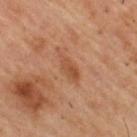The lesion was photographed on a routine skin check and not biopsied; there is no pathology result.
A male patient, in their mid- to late 50s.
The total-body-photography lesion software estimated a lesion area of about 5.5 mm², a shape eccentricity near 0.9, and a symmetry-axis asymmetry near 0.2. The software also gave a mean CIELAB color near L≈49 a*≈23 b*≈34, about 8 CIELAB-L* units darker than the surrounding skin, and a normalized lesion–skin contrast near 6. The analysis additionally found a border-irregularity index near 3/10, a color-variation rating of about 3/10, and a peripheral color-asymmetry measure near 1. The software also gave lesion-presence confidence of about 100/100.
A region of skin cropped from a whole-body photographic capture, roughly 15 mm wide.
Approximately 4 mm at its widest.
On the upper back.
Captured under cross-polarized illumination.